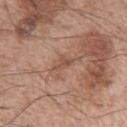Image and clinical context:
The lesion is on the left upper arm. Measured at roughly 3 mm in maximum diameter. A male patient aged around 65. This is a white-light tile. A roughly 15 mm field-of-view crop from a total-body skin photograph. The total-body-photography lesion software estimated a lesion area of about 3 mm², an outline eccentricity of about 0.8 (0 = round, 1 = elongated), and two-axis asymmetry of about 0.35. And it measured border irregularity of about 4 on a 0–10 scale, a within-lesion color-variation index near 1/10, and radial color variation of about 0.5. The analysis additionally found an automated nevus-likeness rating near 0 out of 100.